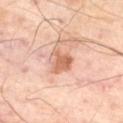The lesion was photographed on a routine skin check and not biopsied; there is no pathology result. Captured under cross-polarized illumination. A 15 mm close-up extracted from a 3D total-body photography capture. Automated tile analysis of the lesion measured a lesion area of about 5.5 mm² and an eccentricity of roughly 0.6. From the right thigh. The subject is a male aged approximately 70. Longest diameter approximately 3 mm.